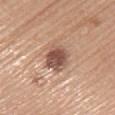Acquisition and patient details:
Measured at roughly 3.5 mm in maximum diameter. From the back. A female subject aged approximately 65. A close-up tile cropped from a whole-body skin photograph, about 15 mm across. This is a white-light tile.